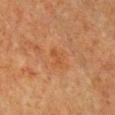Case summary:
* subject — male, aged 63–67
* lesion diameter — ≈2.5 mm
* image — ~15 mm crop, total-body skin-cancer survey
* illumination — cross-polarized illumination
* TBP lesion metrics — an average lesion color of about L≈42 a*≈23 b*≈34 (CIELAB), a lesion–skin lightness drop of about 5, and a lesion-to-skin contrast of about 5 (normalized; higher = more distinct); a nevus-likeness score of about 0/100 and a lesion-detection confidence of about 100/100
* body site — the chest